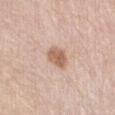notes: total-body-photography surveillance lesion; no biopsy | imaging modality: 15 mm crop, total-body photography | illumination: white-light illumination | subject: male, approximately 65 years of age | lesion size: about 3 mm | location: the abdomen.